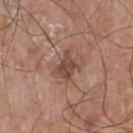Notes:
– biopsy status · imaged on a skin check; not biopsied
– anatomic site · the chest
– lighting · white-light
– image-analysis metrics · a footprint of about 5.5 mm², an eccentricity of roughly 0.7, and a shape-asymmetry score of about 0.35 (0 = symmetric); a mean CIELAB color near L≈46 a*≈18 b*≈26, roughly 10 lightness units darker than nearby skin, and a normalized lesion–skin contrast near 8
– subject · male, in their mid- to late 60s
– lesion diameter · about 3 mm
– image source · total-body-photography crop, ~15 mm field of view